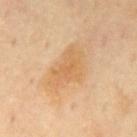Clinical impression:
Recorded during total-body skin imaging; not selected for excision or biopsy.
Clinical summary:
A 15 mm close-up tile from a total-body photography series done for melanoma screening. The lesion's longest dimension is about 6 mm. Automated image analysis of the tile measured about 7 CIELAB-L* units darker than the surrounding skin. And it measured a border-irregularity rating of about 3.5/10, a color-variation rating of about 3.5/10, and radial color variation of about 1. A male patient, aged approximately 70. The lesion is on the back.